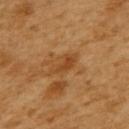biopsy status: no biopsy performed (imaged during a skin exam) | image: total-body-photography crop, ~15 mm field of view | patient: female, aged 53 to 57 | tile lighting: cross-polarized | image-analysis metrics: an area of roughly 5 mm², an eccentricity of roughly 0.85, and a shape-asymmetry score of about 0.3 (0 = symmetric); a border-irregularity rating of about 3/10 and peripheral color asymmetry of about 0.5; an automated nevus-likeness rating near 0 out of 100 and a lesion-detection confidence of about 100/100 | lesion diameter: about 3.5 mm | site: the upper back.